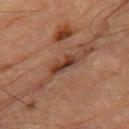Part of a total-body skin-imaging series; this lesion was reviewed on a skin check and was not flagged for biopsy.